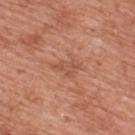Q: Was this lesion biopsied?
A: total-body-photography surveillance lesion; no biopsy
Q: Lesion size?
A: ≈3.5 mm
Q: What are the patient's age and sex?
A: male, aged 68–72
Q: Lesion location?
A: the upper back
Q: What is the imaging modality?
A: ~15 mm crop, total-body skin-cancer survey
Q: What did automated image analysis measure?
A: a mean CIELAB color near L≈54 a*≈25 b*≈32 and a lesion–skin lightness drop of about 7; border irregularity of about 5.5 on a 0–10 scale, a within-lesion color-variation index near 1.5/10, and a peripheral color-asymmetry measure near 0.5; an automated nevus-likeness rating near 0 out of 100 and a detector confidence of about 100 out of 100 that the crop contains a lesion